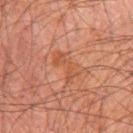On the chest. A male patient, aged approximately 60. A 15 mm crop from a total-body photograph taken for skin-cancer surveillance.